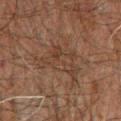notes = no biopsy performed (imaged during a skin exam); acquisition = 15 mm crop, total-body photography; patient = male, approximately 60 years of age; tile lighting = cross-polarized; body site = the chest.